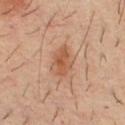Part of a total-body skin-imaging series; this lesion was reviewed on a skin check and was not flagged for biopsy.